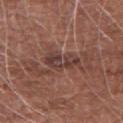Clinical impression: The lesion was tiled from a total-body skin photograph and was not biopsied. Context: The subject is a male aged approximately 65. The recorded lesion diameter is about 4 mm. From the right upper arm. The tile uses white-light illumination. An algorithmic analysis of the crop reported a footprint of about 7 mm², a shape eccentricity near 0.8, and two-axis asymmetry of about 0.35. A close-up tile cropped from a whole-body skin photograph, about 15 mm across.